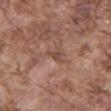follow-up = catalogued during a skin exam; not biopsied
imaging modality = ~15 mm crop, total-body skin-cancer survey
patient = male, aged around 75
illumination = white-light illumination
diameter = ≈2.5 mm
site = the back